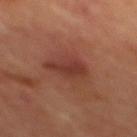| feature | finding |
|---|---|
| follow-up | imaged on a skin check; not biopsied |
| automated metrics | an average lesion color of about L≈35 a*≈24 b*≈26 (CIELAB), a lesion–skin lightness drop of about 8, and a lesion-to-skin contrast of about 7 (normalized; higher = more distinct); border irregularity of about 3.5 on a 0–10 scale, a within-lesion color-variation index near 2/10, and a peripheral color-asymmetry measure near 0.5; an automated nevus-likeness rating near 15 out of 100 and a lesion-detection confidence of about 100/100 |
| size | ~3.5 mm (longest diameter) |
| site | the mid back |
| acquisition | 15 mm crop, total-body photography |
| tile lighting | cross-polarized |
| subject | male, roughly 70 years of age |